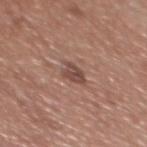Q: Was a biopsy performed?
A: imaged on a skin check; not biopsied
Q: Automated lesion metrics?
A: an average lesion color of about L≈46 a*≈20 b*≈24 (CIELAB) and a normalized border contrast of about 7.5; internal color variation of about 3 on a 0–10 scale
Q: Who is the patient?
A: male, aged 43–47
Q: How was this image acquired?
A: 15 mm crop, total-body photography
Q: Lesion location?
A: the chest
Q: What is the lesion's diameter?
A: ~2.5 mm (longest diameter)
Q: How was the tile lit?
A: white-light illumination